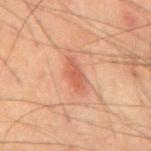follow-up: no biopsy performed (imaged during a skin exam)
illumination: cross-polarized illumination
image: 15 mm crop, total-body photography
subject: male, aged around 65
location: the mid back
lesion size: ~4 mm (longest diameter)